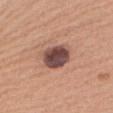Q: Lesion location?
A: the right upper arm
Q: How was this image acquired?
A: 15 mm crop, total-body photography
Q: Lesion size?
A: ≈3.5 mm
Q: Patient demographics?
A: female, approximately 50 years of age